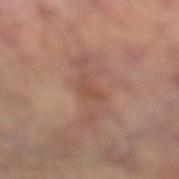Part of a total-body skin-imaging series; this lesion was reviewed on a skin check and was not flagged for biopsy.
Imaged with cross-polarized lighting.
A male subject, aged around 70.
Approximately 2.5 mm at its widest.
On the left lower leg.
The total-body-photography lesion software estimated a lesion area of about 3.5 mm², an eccentricity of roughly 0.8, and two-axis asymmetry of about 0.45. It also reported a lesion color around L≈47 a*≈21 b*≈26 in CIELAB, roughly 6 lightness units darker than nearby skin, and a lesion-to-skin contrast of about 5 (normalized; higher = more distinct). The analysis additionally found a border-irregularity index near 4/10, internal color variation of about 1.5 on a 0–10 scale, and peripheral color asymmetry of about 0.5.
A 15 mm crop from a total-body photograph taken for skin-cancer surveillance.